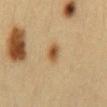{
  "biopsy_status": "not biopsied; imaged during a skin examination",
  "image": {
    "source": "total-body photography crop",
    "field_of_view_mm": 15
  },
  "site": "mid back",
  "patient": {
    "sex": "female",
    "age_approx": 30
  },
  "lesion_size": {
    "long_diameter_mm_approx": 2.5
  }
}